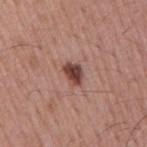Recorded during total-body skin imaging; not selected for excision or biopsy. A region of skin cropped from a whole-body photographic capture, roughly 15 mm wide. A male subject about 55 years old. The lesion-visualizer software estimated a lesion area of about 4.5 mm², an outline eccentricity of about 0.7 (0 = round, 1 = elongated), and a symmetry-axis asymmetry near 0.2. And it measured a lesion color around L≈43 a*≈23 b*≈24 in CIELAB, about 15 CIELAB-L* units darker than the surrounding skin, and a normalized border contrast of about 11. The software also gave border irregularity of about 2 on a 0–10 scale, a within-lesion color-variation index near 5/10, and peripheral color asymmetry of about 1.5. The software also gave an automated nevus-likeness rating near 90 out of 100. This is a white-light tile. The recorded lesion diameter is about 3 mm. Located on the arm.